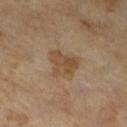No biopsy was performed on this lesion — it was imaged during a full skin examination and was not determined to be concerning. The recorded lesion diameter is about 3.5 mm. A male subject roughly 65 years of age. The lesion-visualizer software estimated an average lesion color of about L≈46 a*≈15 b*≈32 (CIELAB), roughly 8 lightness units darker than nearby skin, and a lesion-to-skin contrast of about 7.5 (normalized; higher = more distinct). The software also gave a classifier nevus-likeness of about 5/100 and a detector confidence of about 100 out of 100 that the crop contains a lesion. A 15 mm close-up tile from a total-body photography series done for melanoma screening.